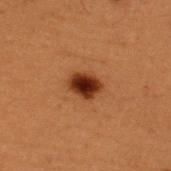Impression: Part of a total-body skin-imaging series; this lesion was reviewed on a skin check and was not flagged for biopsy. Image and clinical context: A male patient, aged approximately 50. On the upper back. The lesion-visualizer software estimated a lesion color around L≈27 a*≈22 b*≈28 in CIELAB and a normalized border contrast of about 13.5. The software also gave a border-irregularity rating of about 1.5/10, a within-lesion color-variation index near 5.5/10, and peripheral color asymmetry of about 1. Captured under cross-polarized illumination. About 3 mm across. A 15 mm close-up tile from a total-body photography series done for melanoma screening.